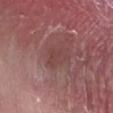follow-up — catalogued during a skin exam; not biopsied
site — the right forearm
image source — ~15 mm crop, total-body skin-cancer survey
size — ~3 mm (longest diameter)
automated metrics — an area of roughly 5 mm² and a shape eccentricity near 0.8
patient — male, about 40 years old
tile lighting — white-light illumination